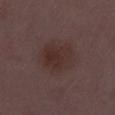• workup — catalogued during a skin exam; not biopsied
• lesion size — ≈4.5 mm
• image source — 15 mm crop, total-body photography
• site — the left thigh
• patient — female, approximately 30 years of age
• automated metrics — a classifier nevus-likeness of about 30/100 and a detector confidence of about 100 out of 100 that the crop contains a lesion
• illumination — white-light